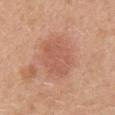notes — imaged on a skin check; not biopsied
acquisition — ~15 mm crop, total-body skin-cancer survey
location — the abdomen
subject — female, aged approximately 40
illumination — white-light illumination
size — about 5 mm
image-analysis metrics — an area of roughly 16 mm² and a shape-asymmetry score of about 0.2 (0 = symmetric); an average lesion color of about L≈57 a*≈25 b*≈31 (CIELAB), about 8 CIELAB-L* units darker than the surrounding skin, and a lesion-to-skin contrast of about 5 (normalized; higher = more distinct); an automated nevus-likeness rating near 30 out of 100 and lesion-presence confidence of about 100/100